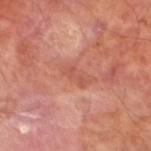Assessment:
The lesion was photographed on a routine skin check and not biopsied; there is no pathology result.
Image and clinical context:
The tile uses cross-polarized illumination. The lesion is located on the left lower leg. This image is a 15 mm lesion crop taken from a total-body photograph. A male subject about 70 years old. Approximately 2.5 mm at its widest.